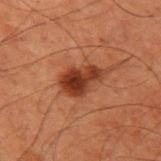The lesion was tiled from a total-body skin photograph and was not biopsied. About 4.5 mm across. From the left upper arm. A region of skin cropped from a whole-body photographic capture, roughly 15 mm wide. A male patient about 60 years old. This is a cross-polarized tile.